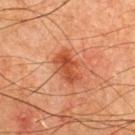Q: Lesion size?
A: ≈4 mm
Q: Automated lesion metrics?
A: a footprint of about 8.5 mm², an eccentricity of roughly 0.8, and two-axis asymmetry of about 0.25; an average lesion color of about L≈44 a*≈29 b*≈35 (CIELAB) and roughly 10 lightness units darker than nearby skin; internal color variation of about 4 on a 0–10 scale
Q: Patient demographics?
A: male, roughly 65 years of age
Q: What lighting was used for the tile?
A: cross-polarized
Q: What kind of image is this?
A: ~15 mm crop, total-body skin-cancer survey
Q: Where on the body is the lesion?
A: the front of the torso